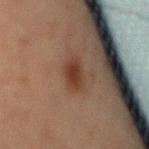Q: Was this lesion biopsied?
A: total-body-photography surveillance lesion; no biopsy
Q: What is the imaging modality?
A: 15 mm crop, total-body photography
Q: Where on the body is the lesion?
A: the abdomen
Q: What are the patient's age and sex?
A: male, aged 63 to 67